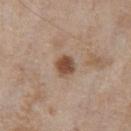Imaged during a routine full-body skin examination; the lesion was not biopsied and no histopathology is available. From the chest. A male subject, aged 63–67. A lesion tile, about 15 mm wide, cut from a 3D total-body photograph.